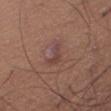The lesion is on the right thigh.
A female subject, aged around 45.
A region of skin cropped from a whole-body photographic capture, roughly 15 mm wide.
The tile uses white-light illumination.